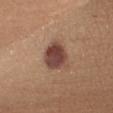The lesion was photographed on a routine skin check and not biopsied; there is no pathology result. This is a white-light tile. A 15 mm crop from a total-body photograph taken for skin-cancer surveillance. A female subject aged approximately 35. Located on the right upper arm. Longest diameter approximately 5 mm.